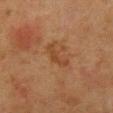Context:
The recorded lesion diameter is about 4 mm. Cropped from a whole-body photographic skin survey; the tile spans about 15 mm. The lesion is on the chest. A female patient, aged approximately 50. An algorithmic analysis of the crop reported an average lesion color of about L≈36 a*≈19 b*≈30 (CIELAB), a lesion–skin lightness drop of about 6, and a normalized lesion–skin contrast near 6. The analysis additionally found a border-irregularity rating of about 5.5/10 and a color-variation rating of about 0/10. The software also gave a nevus-likeness score of about 0/100 and a lesion-detection confidence of about 100/100.